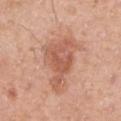Part of a total-body skin-imaging series; this lesion was reviewed on a skin check and was not flagged for biopsy.
On the right upper arm.
Measured at roughly 7.5 mm in maximum diameter.
A 15 mm crop from a total-body photograph taken for skin-cancer surveillance.
Imaged with white-light lighting.
Automated image analysis of the tile measured roughly 10 lightness units darker than nearby skin. And it measured internal color variation of about 4 on a 0–10 scale and radial color variation of about 1. The analysis additionally found a classifier nevus-likeness of about 60/100 and a lesion-detection confidence of about 100/100.
A female subject aged 48–52.